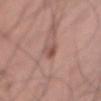The lesion was tiled from a total-body skin photograph and was not biopsied. A male subject, in their mid-50s. A roughly 15 mm field-of-view crop from a total-body skin photograph. The total-body-photography lesion software estimated a mean CIELAB color near L≈51 a*≈21 b*≈24, a lesion–skin lightness drop of about 9, and a normalized lesion–skin contrast near 6.5. The software also gave a classifier nevus-likeness of about 10/100. About 3 mm across. The tile uses white-light illumination. From the abdomen.